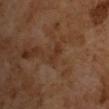Clinical impression: Imaged during a routine full-body skin examination; the lesion was not biopsied and no histopathology is available. Clinical summary: Longest diameter approximately 3 mm. Cropped from a whole-body photographic skin survey; the tile spans about 15 mm. The subject is a male aged approximately 65. The total-body-photography lesion software estimated border irregularity of about 5 on a 0–10 scale, a color-variation rating of about 0/10, and peripheral color asymmetry of about 0. It also reported a classifier nevus-likeness of about 0/100 and a detector confidence of about 100 out of 100 that the crop contains a lesion.